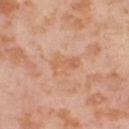biopsy status — imaged on a skin check; not biopsied
site — the right thigh
subject — female, about 55 years old
image source — ~15 mm tile from a whole-body skin photo